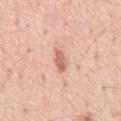  biopsy_status: not biopsied; imaged during a skin examination
  image:
    source: total-body photography crop
    field_of_view_mm: 15
  site: chest
  patient:
    sex: male
    age_approx: 55